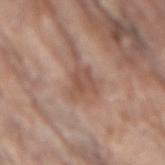The lesion was photographed on a routine skin check and not biopsied; there is no pathology result. A male subject, in their 80s. Approximately 3.5 mm at its widest. The lesion is located on the back. A 15 mm close-up tile from a total-body photography series done for melanoma screening.